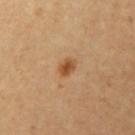Assessment: Imaged during a routine full-body skin examination; the lesion was not biopsied and no histopathology is available. Acquisition and patient details: A roughly 15 mm field-of-view crop from a total-body skin photograph. The subject is a female roughly 30 years of age. The lesion-visualizer software estimated an area of roughly 3.5 mm² and an eccentricity of roughly 0.7. It also reported a mean CIELAB color near L≈51 a*≈23 b*≈39, a lesion–skin lightness drop of about 11, and a normalized border contrast of about 8.5. And it measured a border-irregularity rating of about 2/10, internal color variation of about 4.5 on a 0–10 scale, and a peripheral color-asymmetry measure near 1.5. The lesion is located on the left upper arm. The lesion's longest dimension is about 2.5 mm.